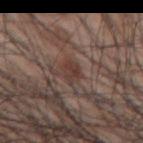{"biopsy_status": "not biopsied; imaged during a skin examination", "patient": {"sex": "male", "age_approx": 70}, "image": {"source": "total-body photography crop", "field_of_view_mm": 15}, "site": "back"}